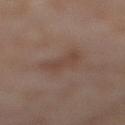Q: Is there a histopathology result?
A: no biopsy performed (imaged during a skin exam)
Q: Where on the body is the lesion?
A: the right lower leg
Q: What is the imaging modality?
A: total-body-photography crop, ~15 mm field of view
Q: What are the patient's age and sex?
A: female, approximately 55 years of age